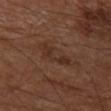Part of a total-body skin-imaging series; this lesion was reviewed on a skin check and was not flagged for biopsy.
The patient is a male aged 63 to 67.
Cropped from a whole-body photographic skin survey; the tile spans about 15 mm.
The lesion's longest dimension is about 5.5 mm.
The tile uses cross-polarized illumination.
The lesion is on the left thigh.